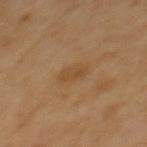Recorded during total-body skin imaging; not selected for excision or biopsy. The lesion-visualizer software estimated a lesion area of about 4 mm², a shape eccentricity near 0.85, and two-axis asymmetry of about 0.25. It also reported a normalized lesion–skin contrast near 5.5. The analysis additionally found a border-irregularity index near 2/10, a color-variation rating of about 1.5/10, and radial color variation of about 0.5. And it measured an automated nevus-likeness rating near 0 out of 100. The recorded lesion diameter is about 3 mm. Imaged with cross-polarized lighting. From the upper back. The patient is a male aged approximately 65. A close-up tile cropped from a whole-body skin photograph, about 15 mm across.